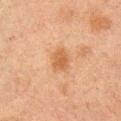image-analysis metrics = a lesion–skin lightness drop of about 8 and a normalized border contrast of about 7; a border-irregularity index near 2/10 and radial color variation of about 1; a nevus-likeness score of about 45/100 | patient = male, aged 48 to 52 | anatomic site = the right upper arm | image = ~15 mm tile from a whole-body skin photo | size = about 3 mm.